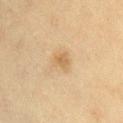  biopsy_status: not biopsied; imaged during a skin examination
  image:
    source: total-body photography crop
    field_of_view_mm: 15
  patient:
    sex: female
    age_approx: 60
  site: chest